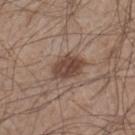Q: Was a biopsy performed?
A: imaged on a skin check; not biopsied
Q: What is the imaging modality?
A: 15 mm crop, total-body photography
Q: How large is the lesion?
A: ≈4 mm
Q: Patient demographics?
A: male, aged 43–47
Q: Where on the body is the lesion?
A: the leg